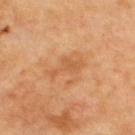The lesion was photographed on a routine skin check and not biopsied; there is no pathology result.